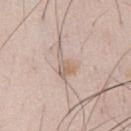Acquisition and patient details:
Longest diameter approximately 4 mm. On the front of the torso. A 15 mm crop from a total-body photograph taken for skin-cancer surveillance. The patient is a male roughly 35 years of age.